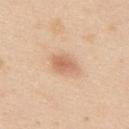Notes:
* workup: no biopsy performed (imaged during a skin exam)
* size: about 3 mm
* anatomic site: the back
* subject: male, in their mid-20s
* acquisition: 15 mm crop, total-body photography
* tile lighting: white-light illumination